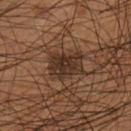Q: Was a biopsy performed?
A: total-body-photography surveillance lesion; no biopsy
Q: What is the imaging modality?
A: total-body-photography crop, ~15 mm field of view
Q: Who is the patient?
A: male, about 55 years old
Q: Lesion location?
A: the left lower leg
Q: What lighting was used for the tile?
A: cross-polarized illumination
Q: What did automated image analysis measure?
A: a lesion area of about 11 mm², a shape eccentricity near 0.7, and two-axis asymmetry of about 0.25
Q: Lesion size?
A: ~4 mm (longest diameter)